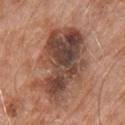This lesion was catalogued during total-body skin photography and was not selected for biopsy. Captured under white-light illumination. The lesion is on the chest. A region of skin cropped from a whole-body photographic capture, roughly 15 mm wide. The subject is a male aged approximately 80.